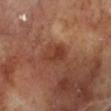{
  "biopsy_status": "not biopsied; imaged during a skin examination",
  "patient": {
    "sex": "male",
    "age_approx": 70
  },
  "image": {
    "source": "total-body photography crop",
    "field_of_view_mm": 15
  },
  "lighting": "cross-polarized",
  "site": "left lower leg",
  "lesion_size": {
    "long_diameter_mm_approx": 3.5
  }
}